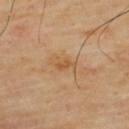{"biopsy_status": "not biopsied; imaged during a skin examination", "patient": {"sex": "male", "age_approx": 65}, "site": "upper back", "image": {"source": "total-body photography crop", "field_of_view_mm": 15}}